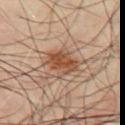biopsy status: total-body-photography surveillance lesion; no biopsy
subject: male, about 50 years old
lighting: cross-polarized
body site: the chest
diameter: about 4 mm
imaging modality: ~15 mm crop, total-body skin-cancer survey